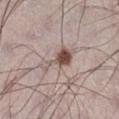Recorded during total-body skin imaging; not selected for excision or biopsy. The lesion is on the right lower leg. Automated tile analysis of the lesion measured a shape-asymmetry score of about 0.55 (0 = symmetric). It also reported a border-irregularity rating of about 7/10 and radial color variation of about 1. Longest diameter approximately 5 mm. Imaged with white-light lighting. A 15 mm crop from a total-body photograph taken for skin-cancer surveillance. The subject is a male in their mid- to late 30s.